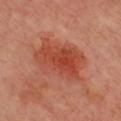Captured during whole-body skin photography for melanoma surveillance; the lesion was not biopsied. A 15 mm close-up tile from a total-body photography series done for melanoma screening. About 7 mm across. Located on the chest. The patient is a female aged 53–57.